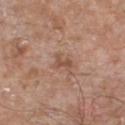follow-up: total-body-photography surveillance lesion; no biopsy
patient: male, aged 63–67
image source: ~15 mm tile from a whole-body skin photo
TBP lesion metrics: a classifier nevus-likeness of about 0/100 and lesion-presence confidence of about 100/100
size: about 2.5 mm
site: the chest